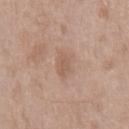Clinical impression: Part of a total-body skin-imaging series; this lesion was reviewed on a skin check and was not flagged for biopsy. Acquisition and patient details: A 15 mm crop from a total-body photograph taken for skin-cancer surveillance. The subject is a male aged around 50. The lesion is located on the chest.